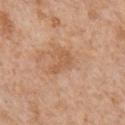Clinical impression:
Captured during whole-body skin photography for melanoma surveillance; the lesion was not biopsied.
Image and clinical context:
A 15 mm close-up extracted from a 3D total-body photography capture. A male patient in their mid-60s. The lesion is located on the chest.